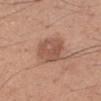workup: no biopsy performed (imaged during a skin exam)
lesion size: ~4 mm (longest diameter)
site: the right forearm
patient: female, approximately 40 years of age
acquisition: 15 mm crop, total-body photography
tile lighting: white-light